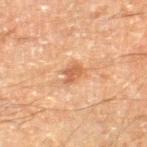The subject is a male aged 58–62. The tile uses cross-polarized illumination. A 15 mm close-up tile from a total-body photography series done for melanoma screening. From the right thigh. The lesion-visualizer software estimated a footprint of about 3.5 mm², an outline eccentricity of about 0.75 (0 = round, 1 = elongated), and a symmetry-axis asymmetry near 0.35. The recorded lesion diameter is about 2.5 mm.